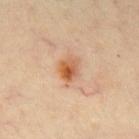The lesion was tiled from a total-body skin photograph and was not biopsied.
A region of skin cropped from a whole-body photographic capture, roughly 15 mm wide.
The lesion is located on the chest.
The subject is a male aged 53 to 57.